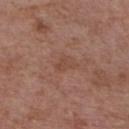Q: Was a biopsy performed?
A: total-body-photography surveillance lesion; no biopsy
Q: What are the patient's age and sex?
A: male, in their 60s
Q: Lesion location?
A: the chest
Q: What kind of image is this?
A: ~15 mm crop, total-body skin-cancer survey
Q: What is the lesion's diameter?
A: about 3 mm